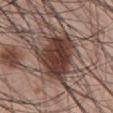biopsy_status: not biopsied; imaged during a skin examination
image:
  source: total-body photography crop
  field_of_view_mm: 15
lighting: white-light
lesion_size:
  long_diameter_mm_approx: 6.0
patient:
  sex: male
  age_approx: 45
site: abdomen
automated_metrics:
  cielab_L: 38
  cielab_a: 18
  cielab_b: 22
  vs_skin_darker_L: 15.0
  vs_skin_contrast_norm: 12.0
  nevus_likeness_0_100: 95
  lesion_detection_confidence_0_100: 100